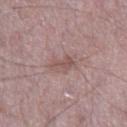{"biopsy_status": "not biopsied; imaged during a skin examination", "patient": {"sex": "male", "age_approx": 65}, "image": {"source": "total-body photography crop", "field_of_view_mm": 15}, "lesion_size": {"long_diameter_mm_approx": 3.0}, "lighting": "white-light", "automated_metrics": {"area_mm2_approx": 4.0, "eccentricity": 0.85, "shape_asymmetry": 0.35, "cielab_L": 52, "cielab_a": 18, "cielab_b": 20, "vs_skin_darker_L": 8.0, "vs_skin_contrast_norm": 6.0, "nevus_likeness_0_100": 0, "lesion_detection_confidence_0_100": 100}, "site": "right thigh"}